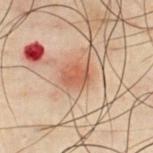workup = no biopsy performed (imaged during a skin exam) | image = ~15 mm crop, total-body skin-cancer survey | body site = the chest | automated metrics = an average lesion color of about L≈46 a*≈21 b*≈29 (CIELAB) and a lesion–skin lightness drop of about 8; a classifier nevus-likeness of about 90/100 and lesion-presence confidence of about 100/100 | lesion size = ~3 mm (longest diameter) | illumination = cross-polarized illumination | patient = male, aged 48–52.